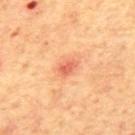Case summary:
• TBP lesion metrics — an area of roughly 4 mm², an eccentricity of roughly 0.7, and two-axis asymmetry of about 0.3; border irregularity of about 2.5 on a 0–10 scale, internal color variation of about 2.5 on a 0–10 scale, and radial color variation of about 1; a classifier nevus-likeness of about 0/100 and lesion-presence confidence of about 100/100
• anatomic site — the mid back
• image source — 15 mm crop, total-body photography
• size — ~2.5 mm (longest diameter)
• subject — male, aged 63 to 67
• lighting — cross-polarized illumination